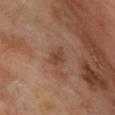lesion diameter — ≈2.5 mm; lighting — cross-polarized illumination; site — the chest; patient — male, about 70 years old; imaging modality — total-body-photography crop, ~15 mm field of view.